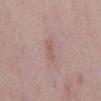Recorded during total-body skin imaging; not selected for excision or biopsy. The total-body-photography lesion software estimated an area of roughly 3 mm², an outline eccentricity of about 0.9 (0 = round, 1 = elongated), and two-axis asymmetry of about 0.25. The lesion is on the back. A female patient in their 50s. Captured under white-light illumination. A 15 mm crop from a total-body photograph taken for skin-cancer surveillance. Approximately 3 mm at its widest.